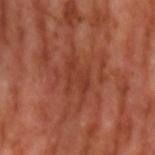Clinical impression:
The lesion was tiled from a total-body skin photograph and was not biopsied.
Acquisition and patient details:
From the left upper arm. Measured at roughly 4.5 mm in maximum diameter. The patient is a male in their mid-50s. A 15 mm crop from a total-body photograph taken for skin-cancer surveillance. Automated image analysis of the tile measured an outline eccentricity of about 0.8 (0 = round, 1 = elongated) and two-axis asymmetry of about 0.4. The tile uses cross-polarized illumination.